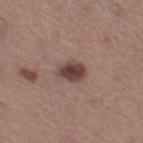The lesion was photographed on a routine skin check and not biopsied; there is no pathology result.
The lesion's longest dimension is about 3 mm.
A region of skin cropped from a whole-body photographic capture, roughly 15 mm wide.
From the right thigh.
A female patient aged 63 to 67.